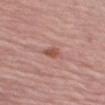Q: Was a biopsy performed?
A: no biopsy performed (imaged during a skin exam)
Q: What did automated image analysis measure?
A: about 10 CIELAB-L* units darker than the surrounding skin and a normalized border contrast of about 7
Q: Who is the patient?
A: male, aged around 65
Q: What is the imaging modality?
A: total-body-photography crop, ~15 mm field of view
Q: How was the tile lit?
A: white-light
Q: Where on the body is the lesion?
A: the arm
Q: Lesion size?
A: ≈3.5 mm